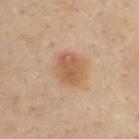<case>
<image>
  <source>total-body photography crop</source>
  <field_of_view_mm>15</field_of_view_mm>
</image>
<lighting>cross-polarized</lighting>
<site>upper back</site>
<patient>
  <sex>male</sex>
  <age_approx>45</age_approx>
</patient>
<lesion_size>
  <long_diameter_mm_approx>3.5</long_diameter_mm_approx>
</lesion_size>
</case>